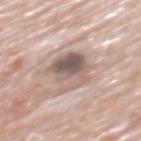A male patient, about 80 years old. The recorded lesion diameter is about 5 mm. This is a white-light tile. Cropped from a whole-body photographic skin survey; the tile spans about 15 mm. The lesion is located on the mid back.